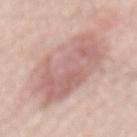{
  "biopsy_status": "not biopsied; imaged during a skin examination",
  "lesion_size": {
    "long_diameter_mm_approx": 11.0
  },
  "lighting": "white-light",
  "image": {
    "source": "total-body photography crop",
    "field_of_view_mm": 15
  },
  "site": "mid back",
  "automated_metrics": {
    "area_mm2_approx": 43.0,
    "eccentricity": 0.9,
    "shape_asymmetry": 0.2,
    "border_irregularity_0_10": 3.0,
    "color_variation_0_10": 5.0,
    "peripheral_color_asymmetry": 1.5
  },
  "patient": {
    "sex": "male",
    "age_approx": 70
  }
}